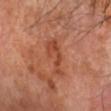Assessment: Captured during whole-body skin photography for melanoma surveillance; the lesion was not biopsied. Acquisition and patient details: The patient is a male in their 70s. A region of skin cropped from a whole-body photographic capture, roughly 15 mm wide. About 4.5 mm across. Located on the left forearm. Automated image analysis of the tile measured a footprint of about 7 mm². The software also gave an average lesion color of about L≈45 a*≈28 b*≈33 (CIELAB), about 8 CIELAB-L* units darker than the surrounding skin, and a lesion-to-skin contrast of about 6 (normalized; higher = more distinct). It also reported a border-irregularity index near 7/10, a color-variation rating of about 2.5/10, and radial color variation of about 1. It also reported a classifier nevus-likeness of about 0/100 and lesion-presence confidence of about 100/100.